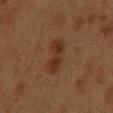Imaged during a routine full-body skin examination; the lesion was not biopsied and no histopathology is available. A female patient, aged 38–42. The lesion is located on the upper back. Imaged with cross-polarized lighting. The recorded lesion diameter is about 4.5 mm. A roughly 15 mm field-of-view crop from a total-body skin photograph.